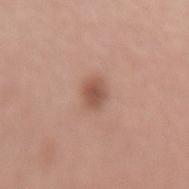Clinical impression:
Imaged during a routine full-body skin examination; the lesion was not biopsied and no histopathology is available.
Acquisition and patient details:
A male patient, approximately 75 years of age. Automated tile analysis of the lesion measured a lesion area of about 5 mm², an eccentricity of roughly 0.65, and a shape-asymmetry score of about 0.1 (0 = symmetric). And it measured a lesion color around L≈53 a*≈22 b*≈28 in CIELAB, roughly 11 lightness units darker than nearby skin, and a normalized border contrast of about 7.5. The software also gave a classifier nevus-likeness of about 80/100 and a lesion-detection confidence of about 100/100. About 3 mm across. A 15 mm close-up extracted from a 3D total-body photography capture. On the lower back.